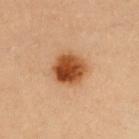The lesion was tiled from a total-body skin photograph and was not biopsied. A close-up tile cropped from a whole-body skin photograph, about 15 mm across. The tile uses cross-polarized illumination. Automated tile analysis of the lesion measured border irregularity of about 1 on a 0–10 scale, a within-lesion color-variation index near 6/10, and radial color variation of about 2. And it measured a nevus-likeness score of about 100/100 and lesion-presence confidence of about 100/100. The lesion is on the chest. A female patient, aged 38 to 42. The recorded lesion diameter is about 3.5 mm.